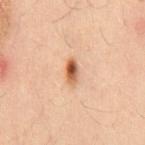workup: total-body-photography surveillance lesion; no biopsy
lesion diameter: ≈3.5 mm
illumination: cross-polarized illumination
location: the mid back
image source: ~15 mm crop, total-body skin-cancer survey
automated lesion analysis: an area of roughly 4 mm², an outline eccentricity of about 0.9 (0 = round, 1 = elongated), and a symmetry-axis asymmetry near 0.25
patient: male, aged 48–52